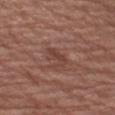biopsy status = imaged on a skin check; not biopsied
image-analysis metrics = an area of roughly 4 mm² and a symmetry-axis asymmetry near 0.3; an average lesion color of about L≈42 a*≈21 b*≈24 (CIELAB), roughly 7 lightness units darker than nearby skin, and a normalized border contrast of about 6; border irregularity of about 4 on a 0–10 scale and internal color variation of about 1 on a 0–10 scale
anatomic site = the left upper arm
lighting = white-light
size = ~3 mm (longest diameter)
subject = male, about 75 years old
image source = ~15 mm tile from a whole-body skin photo